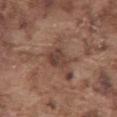Clinical impression: Recorded during total-body skin imaging; not selected for excision or biopsy. Image and clinical context: The lesion is located on the abdomen. About 3 mm across. This is a white-light tile. A male patient, about 75 years old. A roughly 15 mm field-of-view crop from a total-body skin photograph.